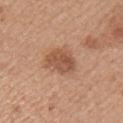Recorded during total-body skin imaging; not selected for excision or biopsy. From the right upper arm. Captured under white-light illumination. This image is a 15 mm lesion crop taken from a total-body photograph. An algorithmic analysis of the crop reported an area of roughly 9 mm², an outline eccentricity of about 0.55 (0 = round, 1 = elongated), and a symmetry-axis asymmetry near 0.2. And it measured roughly 10 lightness units darker than nearby skin and a normalized lesion–skin contrast near 7.5. The software also gave a nevus-likeness score of about 55/100. A female patient, in their mid-30s. The recorded lesion diameter is about 3.5 mm.